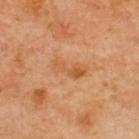Captured during whole-body skin photography for melanoma surveillance; the lesion was not biopsied.
Located on the back.
A 15 mm close-up tile from a total-body photography series done for melanoma screening.
About 4 mm across.
Captured under cross-polarized illumination.
A male patient aged 68 to 72.